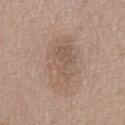Recorded during total-body skin imaging; not selected for excision or biopsy.
The total-body-photography lesion software estimated an area of roughly 19 mm², an eccentricity of roughly 0.85, and a symmetry-axis asymmetry near 0.15. It also reported a border-irregularity index near 2.5/10, internal color variation of about 4 on a 0–10 scale, and peripheral color asymmetry of about 1.5.
A male subject, aged 53–57.
From the back.
A region of skin cropped from a whole-body photographic capture, roughly 15 mm wide.
The recorded lesion diameter is about 6.5 mm.